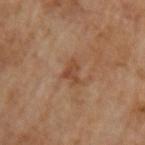No biopsy was performed on this lesion — it was imaged during a full skin examination and was not determined to be concerning. The lesion's longest dimension is about 3 mm. This is a cross-polarized tile. A lesion tile, about 15 mm wide, cut from a 3D total-body photograph. A male subject approximately 65 years of age.